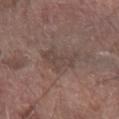This lesion was catalogued during total-body skin photography and was not selected for biopsy. Longest diameter approximately 5 mm. A male subject aged 73 to 77. A roughly 15 mm field-of-view crop from a total-body skin photograph. Imaged with white-light lighting. Located on the left forearm.